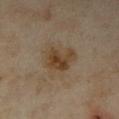| key | value |
|---|---|
| subject | female, aged 33 to 37 |
| site | the right forearm |
| image source | 15 mm crop, total-body photography |
| image-analysis metrics | an eccentricity of roughly 0.6 and a shape-asymmetry score of about 0.35 (0 = symmetric) |
| illumination | cross-polarized |